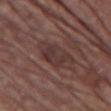| key | value |
|---|---|
| workup | imaged on a skin check; not biopsied |
| tile lighting | white-light illumination |
| image source | ~15 mm tile from a whole-body skin photo |
| subject | male, approximately 85 years of age or older |
| site | the chest |